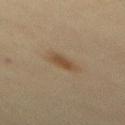{"biopsy_status": "not biopsied; imaged during a skin examination", "lighting": "cross-polarized", "site": "mid back", "patient": {"sex": "female", "age_approx": 45}, "lesion_size": {"long_diameter_mm_approx": 3.0}, "automated_metrics": {"border_irregularity_0_10": 2.5, "color_variation_0_10": 1.5, "peripheral_color_asymmetry": 0.5, "nevus_likeness_0_100": 90, "lesion_detection_confidence_0_100": 100}, "image": {"source": "total-body photography crop", "field_of_view_mm": 15}}